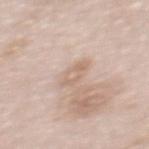Imaged during a routine full-body skin examination; the lesion was not biopsied and no histopathology is available. A female patient, aged around 65. The total-body-photography lesion software estimated a border-irregularity index near 3/10 and a peripheral color-asymmetry measure near 1. The analysis additionally found a classifier nevus-likeness of about 0/100 and a lesion-detection confidence of about 100/100. A roughly 15 mm field-of-view crop from a total-body skin photograph. The tile uses white-light illumination. From the back.